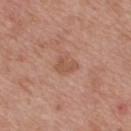workup: total-body-photography surveillance lesion; no biopsy
illumination: white-light illumination
body site: the upper back
imaging modality: 15 mm crop, total-body photography
size: about 3 mm
patient: male, in their mid- to late 60s